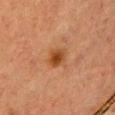Imaged during a routine full-body skin examination; the lesion was not biopsied and no histopathology is available. A lesion tile, about 15 mm wide, cut from a 3D total-body photograph. The subject is a female aged 38–42. Approximately 2.5 mm at its widest. Captured under cross-polarized illumination. The lesion is located on the chest.